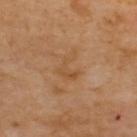Imaged during a routine full-body skin examination; the lesion was not biopsied and no histopathology is available. A 15 mm close-up extracted from a 3D total-body photography capture. Longest diameter approximately 3.5 mm. The lesion is located on the upper back. The tile uses cross-polarized illumination.